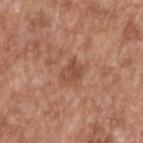Q: Was this lesion biopsied?
A: imaged on a skin check; not biopsied
Q: What is the imaging modality?
A: 15 mm crop, total-body photography
Q: What are the patient's age and sex?
A: male, in their mid-60s
Q: What is the anatomic site?
A: the upper back